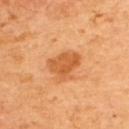| field | value |
|---|---|
| workup | total-body-photography surveillance lesion; no biopsy |
| anatomic site | the upper back |
| lighting | cross-polarized illumination |
| acquisition | 15 mm crop, total-body photography |
| subject | male, in their 60s |
| automated metrics | an average lesion color of about L≈57 a*≈28 b*≈44 (CIELAB), a lesion–skin lightness drop of about 10, and a normalized lesion–skin contrast near 7 |